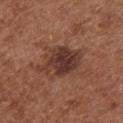Captured during whole-body skin photography for melanoma surveillance; the lesion was not biopsied.
A female patient in their mid- to late 70s.
An algorithmic analysis of the crop reported a lesion area of about 15 mm², an eccentricity of roughly 0.75, and a symmetry-axis asymmetry near 0.25. And it measured an average lesion color of about L≈36 a*≈21 b*≈24 (CIELAB), about 11 CIELAB-L* units darker than the surrounding skin, and a normalized lesion–skin contrast near 10. The analysis additionally found a classifier nevus-likeness of about 30/100 and a detector confidence of about 100 out of 100 that the crop contains a lesion.
Imaged with white-light lighting.
Located on the front of the torso.
A region of skin cropped from a whole-body photographic capture, roughly 15 mm wide.